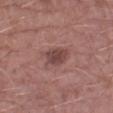| field | value |
|---|---|
| follow-up | no biopsy performed (imaged during a skin exam) |
| subject | male, about 55 years old |
| tile lighting | white-light illumination |
| diameter | about 3.5 mm |
| automated lesion analysis | a lesion area of about 5.5 mm² and a shape eccentricity near 0.8; an average lesion color of about L≈44 a*≈22 b*≈20 (CIELAB); peripheral color asymmetry of about 1 |
| image source | ~15 mm crop, total-body skin-cancer survey |
| body site | the left lower leg |